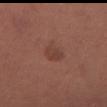No biopsy was performed on this lesion — it was imaged during a full skin examination and was not determined to be concerning. The lesion is on the left leg. Imaged with white-light lighting. Automated image analysis of the tile measured an area of roughly 4 mm² and two-axis asymmetry of about 0.35. The software also gave a classifier nevus-likeness of about 40/100 and a detector confidence of about 100 out of 100 that the crop contains a lesion. Approximately 3 mm at its widest. A female subject, aged 63 to 67. A lesion tile, about 15 mm wide, cut from a 3D total-body photograph.